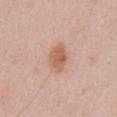Q: Is there a histopathology result?
A: imaged on a skin check; not biopsied
Q: What is the imaging modality?
A: 15 mm crop, total-body photography
Q: What is the anatomic site?
A: the abdomen
Q: How was the tile lit?
A: white-light illumination
Q: Who is the patient?
A: male, in their mid- to late 50s
Q: Automated lesion metrics?
A: an automated nevus-likeness rating near 90 out of 100 and lesion-presence confidence of about 100/100